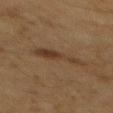The lesion was photographed on a routine skin check and not biopsied; there is no pathology result.